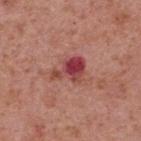<record>
<biopsy_status>not biopsied; imaged during a skin examination</biopsy_status>
<site>upper back</site>
<patient>
  <sex>male</sex>
  <age_approx>70</age_approx>
</patient>
<image>
  <source>total-body photography crop</source>
  <field_of_view_mm>15</field_of_view_mm>
</image>
</record>